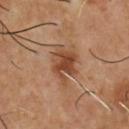Part of a total-body skin-imaging series; this lesion was reviewed on a skin check and was not flagged for biopsy.
Approximately 4 mm at its widest.
The lesion is on the chest.
A male patient roughly 55 years of age.
A 15 mm close-up extracted from a 3D total-body photography capture.
This is a cross-polarized tile.
The total-body-photography lesion software estimated a footprint of about 8 mm², a shape eccentricity near 0.65, and a shape-asymmetry score of about 0.4 (0 = symmetric). The software also gave a mean CIELAB color near L≈43 a*≈22 b*≈32, about 11 CIELAB-L* units darker than the surrounding skin, and a lesion-to-skin contrast of about 9 (normalized; higher = more distinct). It also reported a within-lesion color-variation index near 3/10. The analysis additionally found an automated nevus-likeness rating near 55 out of 100 and lesion-presence confidence of about 100/100.